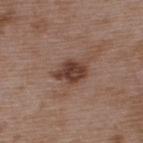<case>
<biopsy_status>not biopsied; imaged during a skin examination</biopsy_status>
<image>
  <source>total-body photography crop</source>
  <field_of_view_mm>15</field_of_view_mm>
</image>
<site>back</site>
<patient>
  <sex>male</sex>
  <age_approx>50</age_approx>
</patient>
<lighting>white-light</lighting>
<lesion_size>
  <long_diameter_mm_approx>3.5</long_diameter_mm_approx>
</lesion_size>
</case>